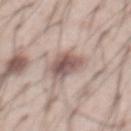biopsy_status: not biopsied; imaged during a skin examination
image:
  source: total-body photography crop
  field_of_view_mm: 15
patient:
  sex: male
  age_approx: 55
site: front of the torso
lighting: white-light
lesion_size:
  long_diameter_mm_approx: 4.5